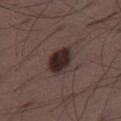Q: Was this lesion biopsied?
A: catalogued during a skin exam; not biopsied
Q: What is the anatomic site?
A: the right thigh
Q: Illumination type?
A: white-light
Q: Automated lesion metrics?
A: a mean CIELAB color near L≈26 a*≈15 b*≈16 and a lesion-to-skin contrast of about 13.5 (normalized; higher = more distinct); a detector confidence of about 100 out of 100 that the crop contains a lesion
Q: What is the imaging modality?
A: total-body-photography crop, ~15 mm field of view
Q: Lesion size?
A: ~4 mm (longest diameter)
Q: What are the patient's age and sex?
A: male, aged around 50